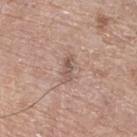Notes:
• follow-up — total-body-photography surveillance lesion; no biopsy
• body site — the left lower leg
• patient — male, approximately 65 years of age
• imaging modality — 15 mm crop, total-body photography